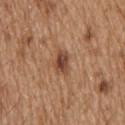Assessment:
Captured during whole-body skin photography for melanoma surveillance; the lesion was not biopsied.
Image and clinical context:
From the head or neck. A male patient aged approximately 70. A 15 mm close-up tile from a total-body photography series done for melanoma screening. About 3 mm across. The tile uses white-light illumination.